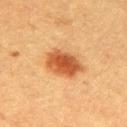Part of a total-body skin-imaging series; this lesion was reviewed on a skin check and was not flagged for biopsy. Located on the mid back. This is a cross-polarized tile. The subject is a female aged around 55. A 15 mm close-up tile from a total-body photography series done for melanoma screening.